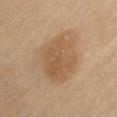Findings:
• follow-up — no biopsy performed (imaged during a skin exam)
• anatomic site — the right upper arm
• size — ≈6.5 mm
• illumination — cross-polarized illumination
• patient — male, roughly 60 years of age
• automated lesion analysis — a lesion area of about 23 mm², a shape eccentricity near 0.6, and two-axis asymmetry of about 0.2
• image source — total-body-photography crop, ~15 mm field of view